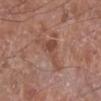| field | value |
|---|---|
| workup | no biopsy performed (imaged during a skin exam) |
| imaging modality | 15 mm crop, total-body photography |
| location | the right lower leg |
| patient | male, aged around 60 |